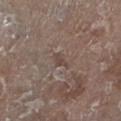The subject is a male in their mid- to late 60s.
An algorithmic analysis of the crop reported an outline eccentricity of about 0.85 (0 = round, 1 = elongated) and two-axis asymmetry of about 0.4. The software also gave an average lesion color of about L≈44 a*≈14 b*≈21 (CIELAB), a lesion–skin lightness drop of about 6, and a normalized lesion–skin contrast near 5.5. The software also gave an automated nevus-likeness rating near 0 out of 100.
A region of skin cropped from a whole-body photographic capture, roughly 15 mm wide.
This is a white-light tile.
About 2.5 mm across.
Located on the left lower leg.